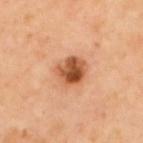The lesion was tiled from a total-body skin photograph and was not biopsied. Longest diameter approximately 3.5 mm. A 15 mm crop from a total-body photograph taken for skin-cancer surveillance. The lesion is on the upper back. This is a cross-polarized tile. A male subject aged 63 to 67.